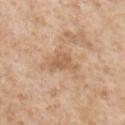Assessment:
Imaged during a routine full-body skin examination; the lesion was not biopsied and no histopathology is available.
Image and clinical context:
A male patient, approximately 65 years of age. Measured at roughly 4 mm in maximum diameter. A 15 mm close-up tile from a total-body photography series done for melanoma screening. Located on the chest. Imaged with white-light lighting.